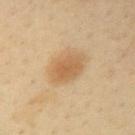This lesion was catalogued during total-body skin photography and was not selected for biopsy. Cropped from a whole-body photographic skin survey; the tile spans about 15 mm. The total-body-photography lesion software estimated a border-irregularity index near 1.5/10, internal color variation of about 3 on a 0–10 scale, and a peripheral color-asymmetry measure near 1. Captured under cross-polarized illumination. The lesion is located on the right upper arm. The patient is a male approximately 40 years of age. The lesion's longest dimension is about 4.5 mm.